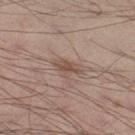{"biopsy_status": "not biopsied; imaged during a skin examination", "image": {"source": "total-body photography crop", "field_of_view_mm": 15}, "patient": {"sex": "male", "age_approx": 30}, "site": "left thigh", "lighting": "white-light", "automated_metrics": {"border_irregularity_0_10": 2.5, "color_variation_0_10": 2.0, "peripheral_color_asymmetry": 0.5}, "lesion_size": {"long_diameter_mm_approx": 3.0}}